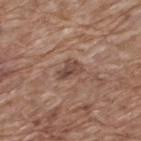{"biopsy_status": "not biopsied; imaged during a skin examination", "automated_metrics": {"cielab_L": 46, "cielab_a": 18, "cielab_b": 25, "vs_skin_darker_L": 9.0, "nevus_likeness_0_100": 0, "lesion_detection_confidence_0_100": 100}, "patient": {"sex": "male", "age_approx": 70}, "site": "back", "lesion_size": {"long_diameter_mm_approx": 3.0}, "lighting": "white-light", "image": {"source": "total-body photography crop", "field_of_view_mm": 15}}